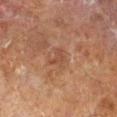Clinical impression:
Captured during whole-body skin photography for melanoma surveillance; the lesion was not biopsied.
Context:
A male subject aged 68–72. Imaged with cross-polarized lighting. A lesion tile, about 15 mm wide, cut from a 3D total-body photograph. The recorded lesion diameter is about 2.5 mm. From the right lower leg.